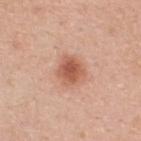{
  "biopsy_status": "not biopsied; imaged during a skin examination",
  "site": "back",
  "patient": {
    "sex": "female",
    "age_approx": 30
  },
  "lighting": "white-light",
  "lesion_size": {
    "long_diameter_mm_approx": 3.0
  },
  "image": {
    "source": "total-body photography crop",
    "field_of_view_mm": 15
  }
}